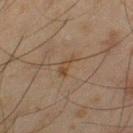Recorded during total-body skin imaging; not selected for excision or biopsy. The subject is a male approximately 45 years of age. Cropped from a total-body skin-imaging series; the visible field is about 15 mm. This is a cross-polarized tile. From the left thigh. Automated tile analysis of the lesion measured a border-irregularity index near 4.5/10 and internal color variation of about 1.5 on a 0–10 scale. And it measured a classifier nevus-likeness of about 0/100 and a lesion-detection confidence of about 100/100. The recorded lesion diameter is about 3 mm.